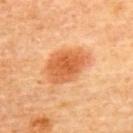| feature | finding |
|---|---|
| follow-up | no biopsy performed (imaged during a skin exam) |
| location | the back |
| subject | female, about 65 years old |
| image | total-body-photography crop, ~15 mm field of view |